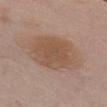notes: total-body-photography surveillance lesion; no biopsy
body site: the chest
lesion diameter: about 6.5 mm
tile lighting: white-light illumination
patient: female, aged approximately 85 or older
image-analysis metrics: an area of roughly 28 mm², an eccentricity of roughly 0.65, and a symmetry-axis asymmetry near 0.15; an average lesion color of about L≈53 a*≈17 b*≈29 (CIELAB), roughly 8 lightness units darker than nearby skin, and a lesion-to-skin contrast of about 7 (normalized; higher = more distinct); an automated nevus-likeness rating near 15 out of 100 and lesion-presence confidence of about 100/100
acquisition: ~15 mm tile from a whole-body skin photo